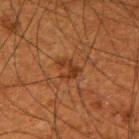patient:
  sex: male
  age_approx: 50
automated_metrics:
  eccentricity: 0.7
  shape_asymmetry: 0.55
  cielab_L: 29
  cielab_a: 21
  cielab_b: 29
  vs_skin_darker_L: 7.0
  vs_skin_contrast_norm: 7.0
  border_irregularity_0_10: 7.5
  color_variation_0_10: 0.0
  peripheral_color_asymmetry: 0.0
  nevus_likeness_0_100: 0
lighting: cross-polarized
image:
  source: total-body photography crop
  field_of_view_mm: 15
lesion_size:
  long_diameter_mm_approx: 2.5
site: right upper arm